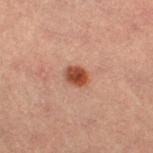– biopsy status — no biopsy performed (imaged during a skin exam)
– anatomic site — the leg
– image — total-body-photography crop, ~15 mm field of view
– tile lighting — cross-polarized
– patient — female, aged around 60
– diameter — about 2.5 mm